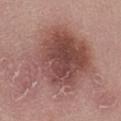follow-up = total-body-photography surveillance lesion; no biopsy
patient = female, aged 53 to 57
site = the abdomen
image = ~15 mm crop, total-body skin-cancer survey